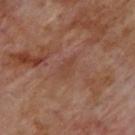<record>
  <biopsy_status>not biopsied; imaged during a skin examination</biopsy_status>
  <lesion_size>
    <long_diameter_mm_approx>2.5</long_diameter_mm_approx>
  </lesion_size>
  <patient>
    <sex>male</sex>
    <age_approx>70</age_approx>
  </patient>
  <site>upper back</site>
  <image>
    <source>total-body photography crop</source>
    <field_of_view_mm>15</field_of_view_mm>
  </image>
  <lighting>cross-polarized</lighting>
</record>